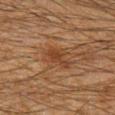| field | value |
|---|---|
| follow-up | catalogued during a skin exam; not biopsied |
| lighting | cross-polarized illumination |
| anatomic site | the left forearm |
| diameter | about 3.5 mm |
| subject | male, approximately 35 years of age |
| imaging modality | total-body-photography crop, ~15 mm field of view |
| image-analysis metrics | a lesion color around L≈31 a*≈18 b*≈27 in CIELAB and a normalized border contrast of about 6; a border-irregularity rating of about 4/10, internal color variation of about 2 on a 0–10 scale, and a peripheral color-asymmetry measure near 0.5 |